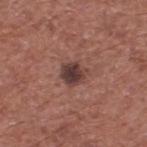Impression: The lesion was tiled from a total-body skin photograph and was not biopsied. Image and clinical context: Automated image analysis of the tile measured a lesion area of about 6.5 mm². And it measured a within-lesion color-variation index near 4/10 and peripheral color asymmetry of about 1. The lesion is located on the upper back. A 15 mm close-up tile from a total-body photography series done for melanoma screening. This is a white-light tile. A male subject aged 73 to 77.